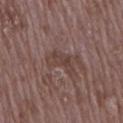TBP lesion metrics=an area of roughly 5.5 mm², a shape eccentricity near 0.85, and a symmetry-axis asymmetry near 0.5; an average lesion color of about L≈40 a*≈17 b*≈21 (CIELAB), roughly 7 lightness units darker than nearby skin, and a normalized border contrast of about 6; a border-irregularity index near 5.5/10, a color-variation rating of about 2.5/10, and peripheral color asymmetry of about 1; a classifier nevus-likeness of about 0/100 and lesion-presence confidence of about 65/100
subject=female, about 70 years old
site=the right upper arm
image source=~15 mm crop, total-body skin-cancer survey